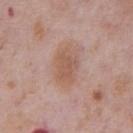Findings:
• biopsy status: total-body-photography surveillance lesion; no biopsy
• image source: ~15 mm tile from a whole-body skin photo
• lighting: white-light illumination
• patient: male, roughly 75 years of age
• location: the front of the torso
• TBP lesion metrics: an area of roughly 10 mm² and a shape-asymmetry score of about 0.2 (0 = symmetric); a border-irregularity rating of about 2/10, a within-lesion color-variation index near 2.5/10, and radial color variation of about 1; a nevus-likeness score of about 5/100 and a detector confidence of about 100 out of 100 that the crop contains a lesion